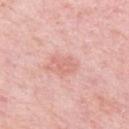Notes:
• follow-up · catalogued during a skin exam; not biopsied